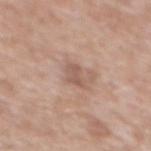Clinical impression:
This lesion was catalogued during total-body skin photography and was not selected for biopsy.
Clinical summary:
The lesion is located on the front of the torso. The patient is a male about 60 years old. Cropped from a whole-body photographic skin survey; the tile spans about 15 mm.